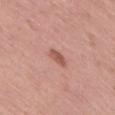Imaged during a routine full-body skin examination; the lesion was not biopsied and no histopathology is available. The lesion is on the right thigh. A female subject, about 55 years old. An algorithmic analysis of the crop reported a lesion–skin lightness drop of about 10. The analysis additionally found a border-irregularity index near 1.5/10, a within-lesion color-variation index near 2.5/10, and a peripheral color-asymmetry measure near 1. This image is a 15 mm lesion crop taken from a total-body photograph.